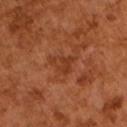This lesion was catalogued during total-body skin photography and was not selected for biopsy. A roughly 15 mm field-of-view crop from a total-body skin photograph. A male subject approximately 65 years of age.